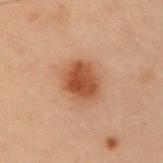<case>
<biopsy_status>not biopsied; imaged during a skin examination</biopsy_status>
<site>right upper arm</site>
<patient>
  <sex>male</sex>
  <age_approx>55</age_approx>
</patient>
<lesion_size>
  <long_diameter_mm_approx>4.5</long_diameter_mm_approx>
</lesion_size>
<lighting>cross-polarized</lighting>
<image>
  <source>total-body photography crop</source>
  <field_of_view_mm>15</field_of_view_mm>
</image>
</case>